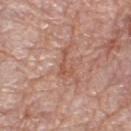Impression: Recorded during total-body skin imaging; not selected for excision or biopsy. Context: A female subject aged 68 to 72. The total-body-photography lesion software estimated a lesion color around L≈54 a*≈23 b*≈30 in CIELAB and a lesion–skin lightness drop of about 7. And it measured a nevus-likeness score of about 0/100. A 15 mm close-up tile from a total-body photography series done for melanoma screening. This is a white-light tile. The lesion is on the right lower leg. The lesion's longest dimension is about 3.5 mm.